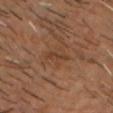{"biopsy_status": "not biopsied; imaged during a skin examination", "patient": {"sex": "male", "age_approx": 50}, "lighting": "cross-polarized", "automated_metrics": {"area_mm2_approx": 2.0, "eccentricity": 0.95, "shape_asymmetry": 0.4, "cielab_L": 39, "cielab_a": 20, "cielab_b": 30, "vs_skin_contrast_norm": 5.5}, "site": "head or neck", "image": {"source": "total-body photography crop", "field_of_view_mm": 15}, "lesion_size": {"long_diameter_mm_approx": 3.0}}